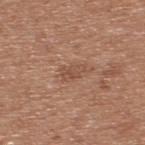Impression:
The lesion was photographed on a routine skin check and not biopsied; there is no pathology result.
Clinical summary:
The lesion is on the upper back. The subject is a male aged 53 to 57. The lesion-visualizer software estimated a lesion area of about 3 mm², an outline eccentricity of about 0.8 (0 = round, 1 = elongated), and a shape-asymmetry score of about 0.4 (0 = symmetric). The analysis additionally found a color-variation rating of about 1.5/10 and a peripheral color-asymmetry measure near 0.5. The analysis additionally found a nevus-likeness score of about 0/100 and a lesion-detection confidence of about 100/100. Approximately 2.5 mm at its widest. This is a white-light tile. A lesion tile, about 15 mm wide, cut from a 3D total-body photograph.